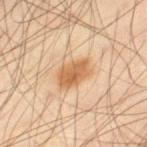Impression: The lesion was photographed on a routine skin check and not biopsied; there is no pathology result. Clinical summary: On the left thigh. A male subject, in their 40s. A 15 mm close-up tile from a total-body photography series done for melanoma screening.